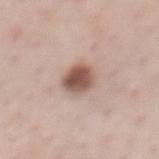{"biopsy_status": "not biopsied; imaged during a skin examination", "patient": {"sex": "male", "age_approx": 60}, "image": {"source": "total-body photography crop", "field_of_view_mm": 15}, "site": "chest", "lesion_size": {"long_diameter_mm_approx": 3.5}, "lighting": "white-light", "automated_metrics": {"vs_skin_darker_L": 16.0, "border_irregularity_0_10": 1.0, "color_variation_0_10": 4.5, "peripheral_color_asymmetry": 1.5, "nevus_likeness_0_100": 100, "lesion_detection_confidence_0_100": 100}}